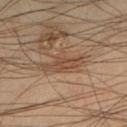Q: Is there a histopathology result?
A: catalogued during a skin exam; not biopsied
Q: Illumination type?
A: cross-polarized
Q: What kind of image is this?
A: ~15 mm crop, total-body skin-cancer survey
Q: What is the lesion's diameter?
A: about 5.5 mm
Q: Patient demographics?
A: male, in their mid- to late 40s
Q: What is the anatomic site?
A: the left lower leg